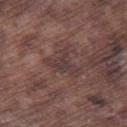Assessment: Captured during whole-body skin photography for melanoma surveillance; the lesion was not biopsied. Context: Cropped from a whole-body photographic skin survey; the tile spans about 15 mm. The lesion is located on the leg. The subject is a male roughly 75 years of age.